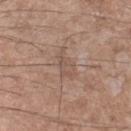• workup · total-body-photography surveillance lesion; no biopsy
• patient · male, aged around 50
• size · ≈4 mm
• site · the left forearm
• illumination · white-light
• image · total-body-photography crop, ~15 mm field of view
• automated lesion analysis · a lesion area of about 5.5 mm², an outline eccentricity of about 0.9 (0 = round, 1 = elongated), and two-axis asymmetry of about 0.35; border irregularity of about 4.5 on a 0–10 scale and radial color variation of about 0.5